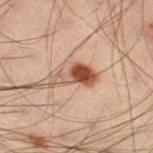Case summary:
* workup: catalogued during a skin exam; not biopsied
* site: the left thigh
* tile lighting: cross-polarized illumination
* acquisition: ~15 mm tile from a whole-body skin photo
* patient: male, roughly 55 years of age